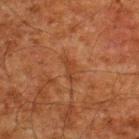biopsy status — no biopsy performed (imaged during a skin exam)
patient — male, roughly 60 years of age
tile lighting — cross-polarized illumination
automated metrics — an average lesion color of about L≈32 a*≈21 b*≈29 (CIELAB), about 5 CIELAB-L* units darker than the surrounding skin, and a normalized lesion–skin contrast near 5; a border-irregularity index near 4.5/10; an automated nevus-likeness rating near 0 out of 100 and lesion-presence confidence of about 100/100
imaging modality — ~15 mm crop, total-body skin-cancer survey
body site — the upper back